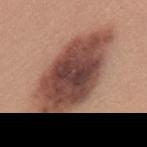<record>
<biopsy_status>not biopsied; imaged during a skin examination</biopsy_status>
<lighting>white-light</lighting>
<image>
  <source>total-body photography crop</source>
  <field_of_view_mm>15</field_of_view_mm>
</image>
<lesion_size>
  <long_diameter_mm_approx>12.0</long_diameter_mm_approx>
</lesion_size>
<site>upper back</site>
<patient>
  <sex>female</sex>
  <age_approx>35</age_approx>
</patient>
<automated_metrics>
  <vs_skin_contrast_norm>12.5</vs_skin_contrast_norm>
  <border_irregularity_0_10>2.5</border_irregularity_0_10>
  <color_variation_0_10>7.0</color_variation_0_10>
  <peripheral_color_asymmetry>2.0</peripheral_color_asymmetry>
  <nevus_likeness_0_100>5</nevus_likeness_0_100>
  <lesion_detection_confidence_0_100>100</lesion_detection_confidence_0_100>
</automated_metrics>
</record>